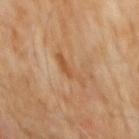Impression: Part of a total-body skin-imaging series; this lesion was reviewed on a skin check and was not flagged for biopsy. Acquisition and patient details: The lesion is on the front of the torso. A 15 mm close-up extracted from a 3D total-body photography capture. A male subject aged 58 to 62.